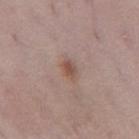Impression: Part of a total-body skin-imaging series; this lesion was reviewed on a skin check and was not flagged for biopsy. Image and clinical context: Automated image analysis of the tile measured a lesion area of about 4 mm², a shape eccentricity near 0.75, and a symmetry-axis asymmetry near 0.2. And it measured border irregularity of about 2 on a 0–10 scale and radial color variation of about 1. The subject is a female aged 28–32. From the left thigh. A roughly 15 mm field-of-view crop from a total-body skin photograph. Imaged with white-light lighting.